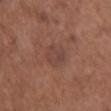{
  "biopsy_status": "not biopsied; imaged during a skin examination",
  "site": "upper back",
  "image": {
    "source": "total-body photography crop",
    "field_of_view_mm": 15
  },
  "patient": {
    "sex": "female",
    "age_approx": 75
  }
}